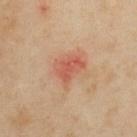The lesion was tiled from a total-body skin photograph and was not biopsied. From the upper back. An algorithmic analysis of the crop reported a lesion color around L≈54 a*≈27 b*≈32 in CIELAB. A female patient approximately 35 years of age. Approximately 3 mm at its widest. A 15 mm crop from a total-body photograph taken for skin-cancer surveillance.